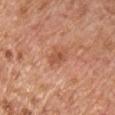This lesion was catalogued during total-body skin photography and was not selected for biopsy. The tile uses white-light illumination. The lesion-visualizer software estimated a footprint of about 3.5 mm². A 15 mm crop from a total-body photograph taken for skin-cancer surveillance. The lesion is on the chest. The recorded lesion diameter is about 3 mm. The subject is a male about 65 years old.